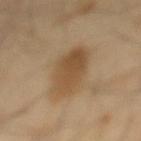Clinical impression:
No biopsy was performed on this lesion — it was imaged during a full skin examination and was not determined to be concerning.
Clinical summary:
This image is a 15 mm lesion crop taken from a total-body photograph. The patient is a male aged around 40. The lesion is on the mid back.